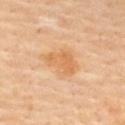Part of a total-body skin-imaging series; this lesion was reviewed on a skin check and was not flagged for biopsy.
A close-up tile cropped from a whole-body skin photograph, about 15 mm across.
The total-body-photography lesion software estimated a border-irregularity rating of about 3.5/10, a within-lesion color-variation index near 2/10, and a peripheral color-asymmetry measure near 0.5. The analysis additionally found a nevus-likeness score of about 10/100.
Longest diameter approximately 4.5 mm.
Captured under cross-polarized illumination.
The lesion is located on the upper back.
A female patient, approximately 60 years of age.